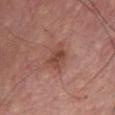Assessment: Imaged during a routine full-body skin examination; the lesion was not biopsied and no histopathology is available.